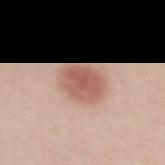  biopsy_status: not biopsied; imaged during a skin examination
  site: mid back
  lesion_size:
    long_diameter_mm_approx: 4.0
  lighting: white-light
  patient:
    sex: female
    age_approx: 40
  image:
    source: total-body photography crop
    field_of_view_mm: 15